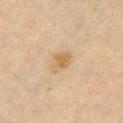Case summary:
– workup · total-body-photography surveillance lesion; no biopsy
– imaging modality · 15 mm crop, total-body photography
– TBP lesion metrics · a footprint of about 5.5 mm², an outline eccentricity of about 0.7 (0 = round, 1 = elongated), and a symmetry-axis asymmetry near 0.25; a border-irregularity index near 2.5/10, a within-lesion color-variation index near 2.5/10, and radial color variation of about 1
– lesion diameter · ≈3 mm
– lighting · cross-polarized illumination
– anatomic site · the chest
– subject · female, aged around 45